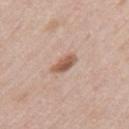The lesion was photographed on a routine skin check and not biopsied; there is no pathology result.
A male patient, in their 50s.
Cropped from a whole-body photographic skin survey; the tile spans about 15 mm.
On the left upper arm.
The lesion's longest dimension is about 3 mm.